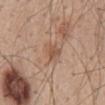Recorded during total-body skin imaging; not selected for excision or biopsy.
This image is a 15 mm lesion crop taken from a total-body photograph.
An algorithmic analysis of the crop reported border irregularity of about 2 on a 0–10 scale, a within-lesion color-variation index near 4/10, and a peripheral color-asymmetry measure near 1.5.
A male patient, about 50 years old.
The lesion's longest dimension is about 2.5 mm.
The lesion is located on the mid back.
This is a white-light tile.